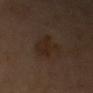The lesion was tiled from a total-body skin photograph and was not biopsied.
Automated image analysis of the tile measured an area of roughly 8 mm². It also reported a lesion color around L≈22 a*≈12 b*≈21 in CIELAB and a lesion–skin lightness drop of about 5.
Captured under cross-polarized illumination.
A male subject approximately 60 years of age.
The recorded lesion diameter is about 4.5 mm.
Cropped from a total-body skin-imaging series; the visible field is about 15 mm.
From the right arm.